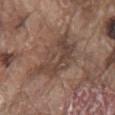image source — 15 mm crop, total-body photography
patient — male, aged approximately 80
lesion diameter — ≈7 mm
body site — the mid back
lighting — white-light
image-analysis metrics — a lesion area of about 18 mm² and a shape-asymmetry score of about 0.3 (0 = symmetric); an average lesion color of about L≈43 a*≈16 b*≈24 (CIELAB), a lesion–skin lightness drop of about 8, and a lesion-to-skin contrast of about 7 (normalized; higher = more distinct); a border-irregularity index near 4.5/10, internal color variation of about 4.5 on a 0–10 scale, and radial color variation of about 1.5